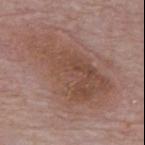Impression:
No biopsy was performed on this lesion — it was imaged during a full skin examination and was not determined to be concerning.
Clinical summary:
Measured at roughly 10.5 mm in maximum diameter. A male patient, aged 63 to 67. On the mid back. A 15 mm close-up tile from a total-body photography series done for melanoma screening. The lesion-visualizer software estimated a footprint of about 37 mm², an eccentricity of roughly 0.9, and a symmetry-axis asymmetry near 0.35. The software also gave a lesion–skin lightness drop of about 8 and a normalized border contrast of about 7. The software also gave border irregularity of about 5.5 on a 0–10 scale, a within-lesion color-variation index near 4.5/10, and radial color variation of about 1.5. The analysis additionally found an automated nevus-likeness rating near 0 out of 100 and a detector confidence of about 100 out of 100 that the crop contains a lesion.